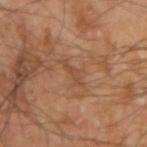This lesion was catalogued during total-body skin photography and was not selected for biopsy. The lesion is on the right upper arm. A male patient roughly 65 years of age. A roughly 15 mm field-of-view crop from a total-body skin photograph.